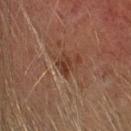Recorded during total-body skin imaging; not selected for excision or biopsy.